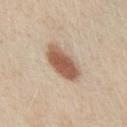Q: Is there a histopathology result?
A: imaged on a skin check; not biopsied
Q: What are the patient's age and sex?
A: male, about 60 years old
Q: How was this image acquired?
A: ~15 mm crop, total-body skin-cancer survey
Q: What is the anatomic site?
A: the chest